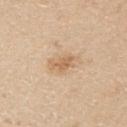biopsy status — no biopsy performed (imaged during a skin exam) | location — the arm | image — total-body-photography crop, ~15 mm field of view | patient — male, aged 38–42.